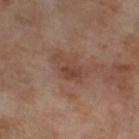This lesion was catalogued during total-body skin photography and was not selected for biopsy.
The total-body-photography lesion software estimated a lesion area of about 6.5 mm², an outline eccentricity of about 0.85 (0 = round, 1 = elongated), and two-axis asymmetry of about 0.3. The analysis additionally found a mean CIELAB color near L≈44 a*≈19 b*≈27 and about 7 CIELAB-L* units darker than the surrounding skin.
The tile uses cross-polarized illumination.
The subject is a female in their mid- to late 50s.
On the leg.
A 15 mm close-up tile from a total-body photography series done for melanoma screening.